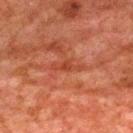workup: imaged on a skin check; not biopsied
image source: total-body-photography crop, ~15 mm field of view
subject: male, approximately 80 years of age
lesion size: about 3.5 mm
anatomic site: the mid back
tile lighting: cross-polarized illumination